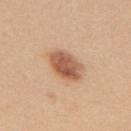Background:
A female subject about 45 years old. A lesion tile, about 15 mm wide, cut from a 3D total-body photograph. The tile uses white-light illumination. The lesion is on the upper back.